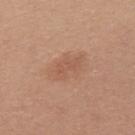Q: Was a biopsy performed?
A: total-body-photography surveillance lesion; no biopsy
Q: What did automated image analysis measure?
A: a lesion area of about 6.5 mm², an eccentricity of roughly 0.8, and a shape-asymmetry score of about 0.35 (0 = symmetric); border irregularity of about 4 on a 0–10 scale and internal color variation of about 2 on a 0–10 scale; a classifier nevus-likeness of about 5/100
Q: Who is the patient?
A: male, approximately 30 years of age
Q: Lesion size?
A: ~4 mm (longest diameter)
Q: What is the imaging modality?
A: ~15 mm crop, total-body skin-cancer survey
Q: What lighting was used for the tile?
A: white-light illumination
Q: Lesion location?
A: the left upper arm